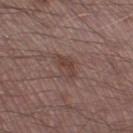biopsy_status: not biopsied; imaged during a skin examination
site: leg
image:
  source: total-body photography crop
  field_of_view_mm: 15
patient:
  sex: male
  age_approx: 70